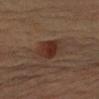Captured during whole-body skin photography for melanoma surveillance; the lesion was not biopsied. This is a cross-polarized tile. The lesion's longest dimension is about 4.5 mm. The patient is a female aged 63 to 67. A close-up tile cropped from a whole-body skin photograph, about 15 mm across. The lesion is on the left upper arm. An algorithmic analysis of the crop reported a footprint of about 13 mm², a shape eccentricity near 0.65, and a symmetry-axis asymmetry near 0.2. The analysis additionally found an average lesion color of about L≈27 a*≈16 b*≈21 (CIELAB) and a normalized border contrast of about 7.5.